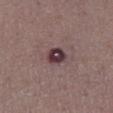{
  "automated_metrics": {
    "area_mm2_approx": 5.5,
    "nevus_likeness_0_100": 0
  },
  "lesion_size": {
    "long_diameter_mm_approx": 2.5
  },
  "image": {
    "source": "total-body photography crop",
    "field_of_view_mm": 15
  },
  "patient": {
    "sex": "male",
    "age_approx": 70
  },
  "lighting": "white-light",
  "site": "chest"
}